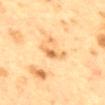Impression:
This lesion was catalogued during total-body skin photography and was not selected for biopsy.
Image and clinical context:
The patient is a female in their 40s. A roughly 15 mm field-of-view crop from a total-body skin photograph. This is a cross-polarized tile. Measured at roughly 3.5 mm in maximum diameter. An algorithmic analysis of the crop reported an average lesion color of about L≈60 a*≈18 b*≈39 (CIELAB) and a normalized lesion–skin contrast near 6.5. The software also gave border irregularity of about 4 on a 0–10 scale and radial color variation of about 1. The analysis additionally found an automated nevus-likeness rating near 0 out of 100 and lesion-presence confidence of about 100/100. From the mid back.